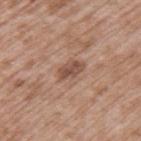Captured during whole-body skin photography for melanoma surveillance; the lesion was not biopsied.
A male patient, about 50 years old.
Located on the upper back.
The tile uses white-light illumination.
This image is a 15 mm lesion crop taken from a total-body photograph.
The recorded lesion diameter is about 3.5 mm.